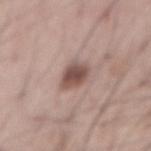{"biopsy_status": "not biopsied; imaged during a skin examination", "lighting": "white-light", "automated_metrics": {"cielab_L": 50, "cielab_a": 19, "cielab_b": 22, "vs_skin_darker_L": 14.0, "vs_skin_contrast_norm": 9.5, "border_irregularity_0_10": 2.0, "color_variation_0_10": 4.0, "peripheral_color_asymmetry": 1.0, "nevus_likeness_0_100": 90}, "site": "abdomen", "patient": {"sex": "male", "age_approx": 55}, "lesion_size": {"long_diameter_mm_approx": 3.5}, "image": {"source": "total-body photography crop", "field_of_view_mm": 15}}